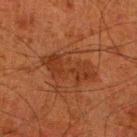Q: Automated lesion metrics?
A: a lesion area of about 13 mm², an eccentricity of roughly 0.9, and two-axis asymmetry of about 0.3; an average lesion color of about L≈29 a*≈22 b*≈29 (CIELAB) and about 6 CIELAB-L* units darker than the surrounding skin; a border-irregularity rating of about 5/10, a within-lesion color-variation index near 2.5/10, and a peripheral color-asymmetry measure near 1; a nevus-likeness score of about 0/100
Q: How was the tile lit?
A: cross-polarized illumination
Q: Lesion location?
A: the leg
Q: What are the patient's age and sex?
A: male, roughly 80 years of age
Q: What kind of image is this?
A: ~15 mm crop, total-body skin-cancer survey
Q: What is the lesion's diameter?
A: ~6.5 mm (longest diameter)